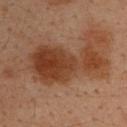biopsy status: imaged on a skin check; not biopsied
subject: male, aged approximately 35
size: about 9.5 mm
body site: the upper back
illumination: cross-polarized illumination
acquisition: total-body-photography crop, ~15 mm field of view